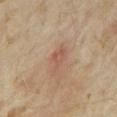<record>
  <biopsy_status>not biopsied; imaged during a skin examination</biopsy_status>
  <patient>
    <sex>female</sex>
    <age_approx>35</age_approx>
  </patient>
  <site>right forearm</site>
  <image>
    <source>total-body photography crop</source>
    <field_of_view_mm>15</field_of_view_mm>
  </image>
</record>